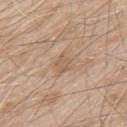* biopsy status — imaged on a skin check; not biopsied
* image-analysis metrics — an eccentricity of roughly 0.75 and a shape-asymmetry score of about 0.25 (0 = symmetric); roughly 6 lightness units darker than nearby skin and a normalized border contrast of about 4.5; a border-irregularity index near 2.5/10, a color-variation rating of about 2/10, and radial color variation of about 0.5; a nevus-likeness score of about 0/100
* illumination — white-light
* size — ~3.5 mm (longest diameter)
* imaging modality — 15 mm crop, total-body photography
* subject — male, aged 78–82
* location — the upper back